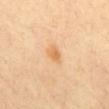Case summary:
– biopsy status · imaged on a skin check; not biopsied
– patient · female, aged 38 to 42
– acquisition · ~15 mm tile from a whole-body skin photo
– diameter · ~2.5 mm (longest diameter)
– body site · the mid back
– tile lighting · cross-polarized illumination